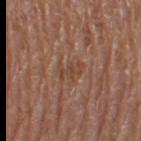Captured during whole-body skin photography for melanoma surveillance; the lesion was not biopsied. Approximately 2.5 mm at its widest. A lesion tile, about 15 mm wide, cut from a 3D total-body photograph. The tile uses white-light illumination. A female subject about 55 years old. From the left thigh.